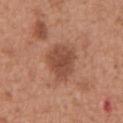Case summary:
• notes — no biopsy performed (imaged during a skin exam)
• subject — male, aged approximately 65
• image source — 15 mm crop, total-body photography
• location — the chest